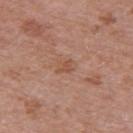No biopsy was performed on this lesion — it was imaged during a full skin examination and was not determined to be concerning. A male subject, roughly 70 years of age. Cropped from a whole-body photographic skin survey; the tile spans about 15 mm. From the right upper arm. The recorded lesion diameter is about 2.5 mm. The total-body-photography lesion software estimated an average lesion color of about L≈52 a*≈22 b*≈30 (CIELAB), a lesion–skin lightness drop of about 6, and a normalized border contrast of about 5. The analysis additionally found a nevus-likeness score of about 0/100 and a lesion-detection confidence of about 100/100. The tile uses white-light illumination.